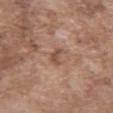Captured during whole-body skin photography for melanoma surveillance; the lesion was not biopsied. The lesion is on the abdomen. A male subject aged around 75. Cropped from a total-body skin-imaging series; the visible field is about 15 mm.